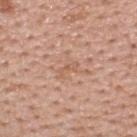On the upper back.
About 2.5 mm across.
A close-up tile cropped from a whole-body skin photograph, about 15 mm across.
A male patient aged around 40.
The tile uses white-light illumination.